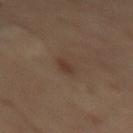follow-up = no biopsy performed (imaged during a skin exam) | location = the right thigh | image = ~15 mm tile from a whole-body skin photo | lighting = cross-polarized | subject = male, aged approximately 70.